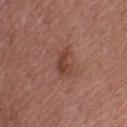biopsy status — imaged on a skin check; not biopsied | diameter — about 2.5 mm | image — total-body-photography crop, ~15 mm field of view | automated metrics — a lesion area of about 4 mm² and an eccentricity of roughly 0.8; border irregularity of about 4 on a 0–10 scale and a within-lesion color-variation index near 1/10 | illumination — white-light illumination | patient — female, aged 38 to 42 | location — the back.